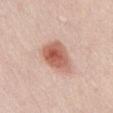Q: Was a biopsy performed?
A: no biopsy performed (imaged during a skin exam)
Q: What is the imaging modality?
A: total-body-photography crop, ~15 mm field of view
Q: What are the patient's age and sex?
A: male, approximately 50 years of age
Q: Automated lesion metrics?
A: a classifier nevus-likeness of about 100/100 and a lesion-detection confidence of about 100/100
Q: Lesion size?
A: ~4.5 mm (longest diameter)
Q: What is the anatomic site?
A: the abdomen
Q: What lighting was used for the tile?
A: white-light illumination